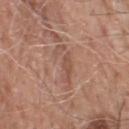The lesion is located on the upper back. Approximately 5 mm at its widest. A 15 mm close-up extracted from a 3D total-body photography capture. Automated image analysis of the tile measured border irregularity of about 6 on a 0–10 scale, a within-lesion color-variation index near 3/10, and radial color variation of about 1. It also reported a nevus-likeness score of about 0/100 and a lesion-detection confidence of about 60/100. This is a white-light tile. The patient is a male approximately 60 years of age.